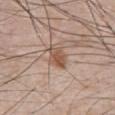{"biopsy_status": "not biopsied; imaged during a skin examination", "lesion_size": {"long_diameter_mm_approx": 3.0}, "automated_metrics": {"area_mm2_approx": 5.5, "eccentricity": 0.7, "shape_asymmetry": 0.2, "border_irregularity_0_10": 2.5, "color_variation_0_10": 2.5, "nevus_likeness_0_100": 75, "lesion_detection_confidence_0_100": 100}, "patient": {"sex": "male", "age_approx": 60}, "lighting": "white-light", "image": {"source": "total-body photography crop", "field_of_view_mm": 15}, "site": "abdomen"}